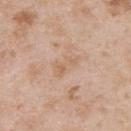Captured during whole-body skin photography for melanoma surveillance; the lesion was not biopsied. This image is a 15 mm lesion crop taken from a total-body photograph. A male patient, in their mid- to late 20s. The lesion is on the upper back. This is a white-light tile. The recorded lesion diameter is about 3.5 mm.